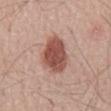notes: imaged on a skin check; not biopsied | image source: 15 mm crop, total-body photography | site: the mid back | patient: male, aged around 70 | automated metrics: a lesion area of about 15 mm² and an outline eccentricity of about 0.8 (0 = round, 1 = elongated); internal color variation of about 4 on a 0–10 scale and radial color variation of about 1; a nevus-likeness score of about 100/100 and a detector confidence of about 100 out of 100 that the crop contains a lesion | illumination: white-light illumination.